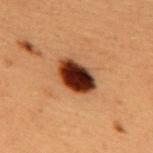The lesion is located on the mid back. A male patient aged approximately 50. A lesion tile, about 15 mm wide, cut from a 3D total-body photograph.